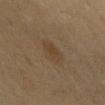Imaged during a routine full-body skin examination; the lesion was not biopsied and no histopathology is available. This image is a 15 mm lesion crop taken from a total-body photograph. From the chest. Automated tile analysis of the lesion measured an area of roughly 5 mm² and a symmetry-axis asymmetry near 0.25. And it measured a mean CIELAB color near L≈37 a*≈13 b*≈28, a lesion–skin lightness drop of about 5, and a normalized border contrast of about 6. The analysis additionally found a within-lesion color-variation index near 1.5/10 and radial color variation of about 0.5. The tile uses cross-polarized illumination. About 3.5 mm across. A male subject, aged approximately 60.